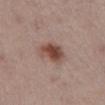Clinical impression:
Captured during whole-body skin photography for melanoma surveillance; the lesion was not biopsied.
Background:
A male patient, about 55 years old. About 3.5 mm across. From the lower back. Imaged with white-light lighting. This image is a 15 mm lesion crop taken from a total-body photograph.